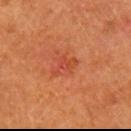follow-up: no biopsy performed (imaged during a skin exam)
patient: male, aged approximately 55
diameter: about 3 mm
illumination: cross-polarized
anatomic site: the right upper arm
image source: total-body-photography crop, ~15 mm field of view